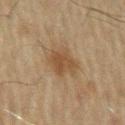Clinical impression:
The lesion was tiled from a total-body skin photograph and was not biopsied.
Acquisition and patient details:
Captured under cross-polarized illumination. A male patient, aged 68 to 72. A close-up tile cropped from a whole-body skin photograph, about 15 mm across. The total-body-photography lesion software estimated a lesion color around L≈39 a*≈14 b*≈29 in CIELAB, about 7 CIELAB-L* units darker than the surrounding skin, and a normalized lesion–skin contrast near 7. The analysis additionally found a nevus-likeness score of about 40/100 and a lesion-detection confidence of about 100/100. From the left upper arm. The recorded lesion diameter is about 3.5 mm.